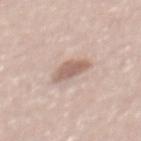A region of skin cropped from a whole-body photographic capture, roughly 15 mm wide.
Approximately 3.5 mm at its widest.
The patient is a male aged 58 to 62.
Captured under white-light illumination.
Automated tile analysis of the lesion measured an automated nevus-likeness rating near 20 out of 100 and a detector confidence of about 100 out of 100 that the crop contains a lesion.
From the mid back.